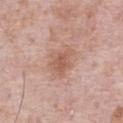The lesion was photographed on a routine skin check and not biopsied; there is no pathology result.
An algorithmic analysis of the crop reported a lesion color around L≈58 a*≈21 b*≈28 in CIELAB, a lesion–skin lightness drop of about 9, and a normalized lesion–skin contrast near 6. And it measured a border-irregularity index near 3.5/10 and a peripheral color-asymmetry measure near 1. And it measured a detector confidence of about 100 out of 100 that the crop contains a lesion.
Cropped from a total-body skin-imaging series; the visible field is about 15 mm.
Longest diameter approximately 4 mm.
Captured under white-light illumination.
A male patient about 70 years old.
On the front of the torso.